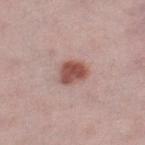No biopsy was performed on this lesion — it was imaged during a full skin examination and was not determined to be concerning. This image is a 15 mm lesion crop taken from a total-body photograph. The tile uses white-light illumination. Automated image analysis of the tile measured a footprint of about 6.5 mm², an eccentricity of roughly 0.6, and a symmetry-axis asymmetry near 0.25. And it measured a lesion color around L≈51 a*≈24 b*≈25 in CIELAB. It also reported an automated nevus-likeness rating near 100 out of 100. A female subject, roughly 45 years of age. From the right lower leg. About 3.5 mm across.